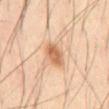  biopsy_status: not biopsied; imaged during a skin examination
  image:
    source: total-body photography crop
    field_of_view_mm: 15
  lesion_size:
    long_diameter_mm_approx: 3.5
  lighting: cross-polarized
  patient:
    sex: male
    age_approx: 60
  site: front of the torso